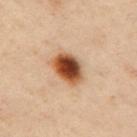follow-up: catalogued during a skin exam; not biopsied | site: the arm | tile lighting: cross-polarized illumination | lesion diameter: ≈4 mm | image: ~15 mm tile from a whole-body skin photo | patient: female, aged approximately 40 | automated lesion analysis: an average lesion color of about L≈52 a*≈25 b*≈37 (CIELAB), roughly 21 lightness units darker than nearby skin, and a lesion-to-skin contrast of about 13.5 (normalized; higher = more distinct); a border-irregularity index near 1.5/10 and radial color variation of about 2.5; a nevus-likeness score of about 100/100 and a lesion-detection confidence of about 100/100.